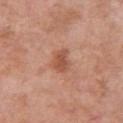Imaged during a routine full-body skin examination; the lesion was not biopsied and no histopathology is available.
A close-up tile cropped from a whole-body skin photograph, about 15 mm across.
A female patient, about 50 years old.
The lesion's longest dimension is about 3 mm.
From the chest.